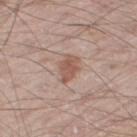Imaged during a routine full-body skin examination; the lesion was not biopsied and no histopathology is available. Longest diameter approximately 3.5 mm. A male patient in their 60s. Automated image analysis of the tile measured an area of roughly 5.5 mm², an eccentricity of roughly 0.75, and a shape-asymmetry score of about 0.25 (0 = symmetric). It also reported a border-irregularity rating of about 3/10 and radial color variation of about 1. It also reported an automated nevus-likeness rating near 80 out of 100 and lesion-presence confidence of about 100/100. The lesion is located on the right thigh. A 15 mm close-up tile from a total-body photography series done for melanoma screening.